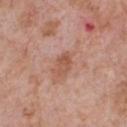notes=total-body-photography surveillance lesion; no biopsy
subject=male, approximately 60 years of age
anatomic site=the chest
acquisition=~15 mm tile from a whole-body skin photo
lesion size=about 2.5 mm
tile lighting=white-light illumination